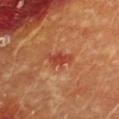Impression:
The lesion was tiled from a total-body skin photograph and was not biopsied.
Background:
Located on the chest. A 15 mm crop from a total-body photograph taken for skin-cancer surveillance. Automated image analysis of the tile measured a lesion area of about 4 mm² and a shape eccentricity near 0.85. The analysis additionally found a lesion–skin lightness drop of about 9. The software also gave border irregularity of about 4 on a 0–10 scale and radial color variation of about 0.5. The subject is a female approximately 80 years of age.